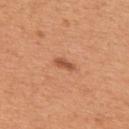Impression: Recorded during total-body skin imaging; not selected for excision or biopsy. Background: This is a white-light tile. On the upper back. The subject is a male aged 53–57. Cropped from a total-body skin-imaging series; the visible field is about 15 mm. The lesion's longest dimension is about 2.5 mm.